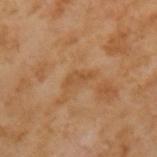notes: catalogued during a skin exam; not biopsied
patient: male, aged 63–67
lesion diameter: ~3.5 mm (longest diameter)
imaging modality: 15 mm crop, total-body photography
lighting: cross-polarized illumination
image-analysis metrics: a lesion area of about 3.5 mm², a shape eccentricity near 0.9, and a symmetry-axis asymmetry near 0.45; a border-irregularity index near 5/10, a color-variation rating of about 1/10, and peripheral color asymmetry of about 0.5; a classifier nevus-likeness of about 0/100 and lesion-presence confidence of about 100/100